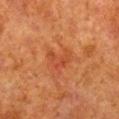• biopsy status: total-body-photography surveillance lesion; no biopsy
• patient: male, about 80 years old
• tile lighting: cross-polarized illumination
• site: the right lower leg
• acquisition: 15 mm crop, total-body photography
• diameter: about 3.5 mm